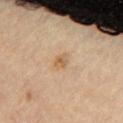Captured during whole-body skin photography for melanoma surveillance; the lesion was not biopsied. The lesion is on the chest. A female subject, roughly 30 years of age. Cropped from a total-body skin-imaging series; the visible field is about 15 mm. The recorded lesion diameter is about 2 mm. The tile uses cross-polarized illumination.